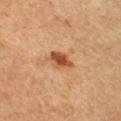Q: Lesion location?
A: the chest
Q: Illumination type?
A: cross-polarized
Q: What are the patient's age and sex?
A: male, aged 63–67
Q: How was this image acquired?
A: ~15 mm crop, total-body skin-cancer survey
Q: What is the lesion's diameter?
A: about 3.5 mm